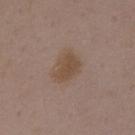- notes — catalogued during a skin exam; not biopsied
- anatomic site — the chest
- subject — female, in their mid- to late 30s
- tile lighting — white-light illumination
- acquisition — 15 mm crop, total-body photography